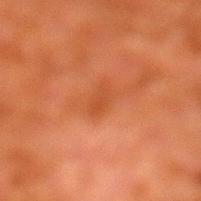The lesion was photographed on a routine skin check and not biopsied; there is no pathology result. A 15 mm close-up extracted from a 3D total-body photography capture. The lesion's longest dimension is about 2.5 mm. The total-body-photography lesion software estimated a shape eccentricity near 0.85 and a symmetry-axis asymmetry near 0.2. The software also gave a lesion color around L≈39 a*≈27 b*≈35 in CIELAB and a lesion-to-skin contrast of about 5 (normalized; higher = more distinct). It also reported a classifier nevus-likeness of about 0/100. The patient is a male roughly 80 years of age. From the left lower leg.